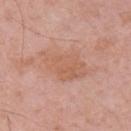Assessment: Captured during whole-body skin photography for melanoma surveillance; the lesion was not biopsied. Acquisition and patient details: A male patient, aged approximately 70. This is a white-light tile. A region of skin cropped from a whole-body photographic capture, roughly 15 mm wide. An algorithmic analysis of the crop reported a lesion color around L≈60 a*≈22 b*≈31 in CIELAB and a lesion-to-skin contrast of about 5.5 (normalized; higher = more distinct). The analysis additionally found a lesion-detection confidence of about 100/100. Measured at roughly 6.5 mm in maximum diameter. The lesion is located on the right upper arm.